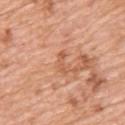| feature | finding |
|---|---|
| follow-up | catalogued during a skin exam; not biopsied |
| diameter | ~2.5 mm (longest diameter) |
| lighting | white-light illumination |
| patient | female, in their mid- to late 40s |
| automated metrics | a lesion area of about 2 mm², a shape eccentricity near 0.9, and two-axis asymmetry of about 0.5; a border-irregularity index near 6/10, a color-variation rating of about 0/10, and peripheral color asymmetry of about 0 |
| body site | the back |
| image | ~15 mm crop, total-body skin-cancer survey |